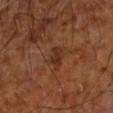| key | value |
|---|---|
| workup | no biopsy performed (imaged during a skin exam) |
| diameter | about 3 mm |
| image source | ~15 mm tile from a whole-body skin photo |
| patient | aged approximately 65 |
| tile lighting | cross-polarized illumination |
| location | the left forearm |